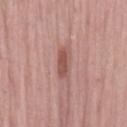Imaged during a routine full-body skin examination; the lesion was not biopsied and no histopathology is available.
The total-body-photography lesion software estimated a footprint of about 5.5 mm² and a symmetry-axis asymmetry near 0.25.
The lesion is located on the left thigh.
A lesion tile, about 15 mm wide, cut from a 3D total-body photograph.
A female patient, aged 63–67.
The tile uses white-light illumination.
Measured at roughly 4 mm in maximum diameter.